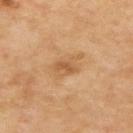Q: Is there a histopathology result?
A: catalogued during a skin exam; not biopsied
Q: What is the imaging modality?
A: 15 mm crop, total-body photography
Q: What are the patient's age and sex?
A: male, aged 53–57
Q: Lesion location?
A: the upper back